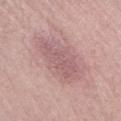Clinical impression:
Part of a total-body skin-imaging series; this lesion was reviewed on a skin check and was not flagged for biopsy.
Clinical summary:
This image is a 15 mm lesion crop taken from a total-body photograph. Longest diameter approximately 6 mm. Automated tile analysis of the lesion measured a lesion color around L≈58 a*≈22 b*≈18 in CIELAB, roughly 8 lightness units darker than nearby skin, and a lesion-to-skin contrast of about 5.5 (normalized; higher = more distinct). And it measured border irregularity of about 3.5 on a 0–10 scale, a color-variation rating of about 2.5/10, and peripheral color asymmetry of about 1. And it measured an automated nevus-likeness rating near 0 out of 100 and a detector confidence of about 80 out of 100 that the crop contains a lesion. Located on the right lower leg. A female patient aged approximately 40. Captured under white-light illumination.